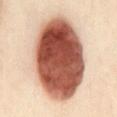Q: Was this lesion biopsied?
A: imaged on a skin check; not biopsied
Q: Where on the body is the lesion?
A: the abdomen
Q: What is the imaging modality?
A: total-body-photography crop, ~15 mm field of view
Q: Patient demographics?
A: female, in their 40s